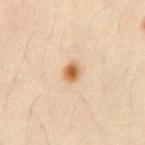workup: no biopsy performed (imaged during a skin exam)
imaging modality: ~15 mm tile from a whole-body skin photo
tile lighting: cross-polarized illumination
body site: the abdomen
lesion diameter: ~2.5 mm (longest diameter)
subject: male, approximately 45 years of age
image-analysis metrics: an eccentricity of roughly 0.6 and a symmetry-axis asymmetry near 0.15; a lesion color around L≈62 a*≈20 b*≈38 in CIELAB, roughly 14 lightness units darker than nearby skin, and a lesion-to-skin contrast of about 10 (normalized; higher = more distinct)